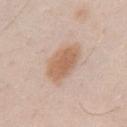workup — no biopsy performed (imaged during a skin exam) | subject — male, about 30 years old | image source — ~15 mm crop, total-body skin-cancer survey | anatomic site — the arm.